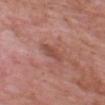Case summary:
• biopsy status · total-body-photography surveillance lesion; no biopsy
• image · total-body-photography crop, ~15 mm field of view
• subject · male, roughly 65 years of age
• anatomic site · the head or neck